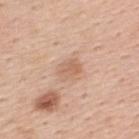notes: total-body-photography surveillance lesion; no biopsy
subject: male, about 55 years old
lighting: white-light illumination
size: about 3 mm
TBP lesion metrics: a lesion area of about 5.5 mm² and an eccentricity of roughly 0.6; a mean CIELAB color near L≈63 a*≈20 b*≈31, a lesion–skin lightness drop of about 8, and a lesion-to-skin contrast of about 5.5 (normalized; higher = more distinct); a border-irregularity rating of about 2/10, internal color variation of about 2.5 on a 0–10 scale, and a peripheral color-asymmetry measure near 1; a classifier nevus-likeness of about 0/100 and a detector confidence of about 100 out of 100 that the crop contains a lesion
site: the back
imaging modality: ~15 mm crop, total-body skin-cancer survey